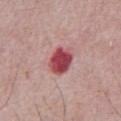Part of a total-body skin-imaging series; this lesion was reviewed on a skin check and was not flagged for biopsy.
This image is a 15 mm lesion crop taken from a total-body photograph.
On the abdomen.
A male patient aged around 65.
Captured under white-light illumination.
About 3.5 mm across.